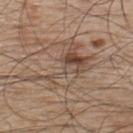Assessment:
Part of a total-body skin-imaging series; this lesion was reviewed on a skin check and was not flagged for biopsy.
Acquisition and patient details:
The lesion's longest dimension is about 7.5 mm. A male subject, about 50 years old. The lesion is located on the upper back. A region of skin cropped from a whole-body photographic capture, roughly 15 mm wide.